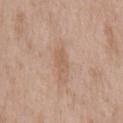Impression:
The lesion was photographed on a routine skin check and not biopsied; there is no pathology result.
Context:
A close-up tile cropped from a whole-body skin photograph, about 15 mm across. Located on the chest. A male subject, aged approximately 50. Approximately 2.5 mm at its widest. The tile uses white-light illumination.